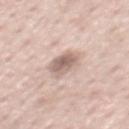Imaged during a routine full-body skin examination; the lesion was not biopsied and no histopathology is available.
The patient is a male in their 60s.
Imaged with white-light lighting.
A lesion tile, about 15 mm wide, cut from a 3D total-body photograph.
The lesion is located on the chest.
Measured at roughly 3.5 mm in maximum diameter.